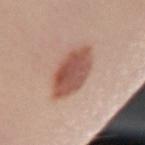Q: Was this lesion biopsied?
A: total-body-photography surveillance lesion; no biopsy
Q: Who is the patient?
A: female, in their mid- to late 20s
Q: Automated lesion metrics?
A: an area of roughly 17 mm², a shape eccentricity near 0.6, and a symmetry-axis asymmetry near 0.1; a mean CIELAB color near L≈53 a*≈23 b*≈27, roughly 14 lightness units darker than nearby skin, and a lesion-to-skin contrast of about 9 (normalized; higher = more distinct)
Q: Where on the body is the lesion?
A: the right forearm
Q: What kind of image is this?
A: ~15 mm crop, total-body skin-cancer survey
Q: What is the lesion's diameter?
A: about 5.5 mm